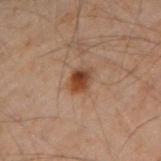Part of a total-body skin-imaging series; this lesion was reviewed on a skin check and was not flagged for biopsy.